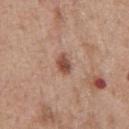  biopsy_status: not biopsied; imaged during a skin examination
  site: chest
  lesion_size:
    long_diameter_mm_approx: 2.5
  patient:
    sex: male
    age_approx: 55
  image:
    source: total-body photography crop
    field_of_view_mm: 15
  automated_metrics:
    area_mm2_approx: 3.5
    eccentricity: 0.75
    shape_asymmetry: 0.25
    cielab_L: 49
    cielab_a: 23
    cielab_b: 28
    vs_skin_darker_L: 13.0
    vs_skin_contrast_norm: 9.5
    nevus_likeness_0_100: 95
    lesion_detection_confidence_0_100: 100